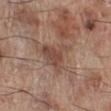Impression: Part of a total-body skin-imaging series; this lesion was reviewed on a skin check and was not flagged for biopsy. Clinical summary: From the right lower leg. Automated tile analysis of the lesion measured a lesion area of about 12 mm² and an eccentricity of roughly 0.65. The analysis additionally found an average lesion color of about L≈48 a*≈19 b*≈25 (CIELAB) and about 9 CIELAB-L* units darker than the surrounding skin. And it measured a lesion-detection confidence of about 95/100. About 5.5 mm across. A close-up tile cropped from a whole-body skin photograph, about 15 mm across. The patient is a male approximately 70 years of age. This is a white-light tile.